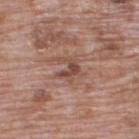Notes:
– workup: total-body-photography surveillance lesion; no biopsy
– imaging modality: ~15 mm tile from a whole-body skin photo
– patient: male, aged 68 to 72
– location: the back
– automated lesion analysis: a lesion color around L≈47 a*≈21 b*≈25 in CIELAB and roughly 9 lightness units darker than nearby skin
– tile lighting: white-light illumination